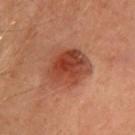Recorded during total-body skin imaging; not selected for excision or biopsy. The lesion's longest dimension is about 5.5 mm. The lesion-visualizer software estimated a footprint of about 19 mm². The software also gave an average lesion color of about L≈38 a*≈25 b*≈28 (CIELAB), roughly 10 lightness units darker than nearby skin, and a normalized lesion–skin contrast near 8.5. The analysis additionally found a border-irregularity index near 2/10 and internal color variation of about 6 on a 0–10 scale. The analysis additionally found lesion-presence confidence of about 100/100. A female patient approximately 50 years of age. Imaged with cross-polarized lighting. Located on the head or neck. A region of skin cropped from a whole-body photographic capture, roughly 15 mm wide.